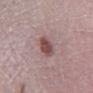• workup · imaged on a skin check; not biopsied
• subject · male, aged approximately 50
• location · the leg
• automated metrics · a shape eccentricity near 0.6; an average lesion color of about L≈48 a*≈21 b*≈20 (CIELAB) and a normalized lesion–skin contrast near 9; a detector confidence of about 100 out of 100 that the crop contains a lesion
• image · total-body-photography crop, ~15 mm field of view